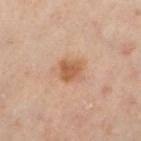Case summary:
* image source · ~15 mm tile from a whole-body skin photo
* lighting · cross-polarized illumination
* anatomic site · the right leg
* automated lesion analysis · an area of roughly 7.5 mm², a shape eccentricity near 0.75, and a shape-asymmetry score of about 0.25 (0 = symmetric); a mean CIELAB color near L≈51 a*≈18 b*≈31 and a normalized border contrast of about 7.5; a color-variation rating of about 3/10 and peripheral color asymmetry of about 1; a classifier nevus-likeness of about 85/100 and a detector confidence of about 100 out of 100 that the crop contains a lesion
* lesion diameter · about 3.5 mm
* patient · female, aged around 60